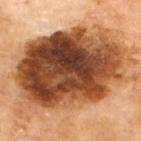Recorded during total-body skin imaging; not selected for excision or biopsy.
Imaged with cross-polarized lighting.
The lesion-visualizer software estimated a shape eccentricity near 0.75 and two-axis asymmetry of about 0.15.
The subject is a male aged 68–72.
From the upper back.
A close-up tile cropped from a whole-body skin photograph, about 15 mm across.